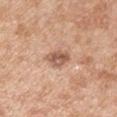Imaged during a routine full-body skin examination; the lesion was not biopsied and no histopathology is available.
On the right upper arm.
Imaged with white-light lighting.
A 15 mm close-up extracted from a 3D total-body photography capture.
A male subject approximately 55 years of age.